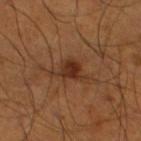Captured during whole-body skin photography for melanoma surveillance; the lesion was not biopsied.
The recorded lesion diameter is about 4 mm.
The lesion is located on the leg.
Captured under cross-polarized illumination.
A male patient in their mid- to late 50s.
An algorithmic analysis of the crop reported a shape eccentricity near 0.65 and a shape-asymmetry score of about 0.45 (0 = symmetric). It also reported a border-irregularity index near 5/10. And it measured an automated nevus-likeness rating near 75 out of 100 and a lesion-detection confidence of about 100/100.
A roughly 15 mm field-of-view crop from a total-body skin photograph.